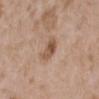The lesion was photographed on a routine skin check and not biopsied; there is no pathology result. A female patient about 35 years old. Cropped from a total-body skin-imaging series; the visible field is about 15 mm. Located on the left upper arm. Captured under white-light illumination. Automated image analysis of the tile measured a footprint of about 5.5 mm², an outline eccentricity of about 0.75 (0 = round, 1 = elongated), and a shape-asymmetry score of about 0.3 (0 = symmetric). And it measured a normalized lesion–skin contrast near 7.5. Measured at roughly 3 mm in maximum diameter.